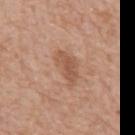* notes: catalogued during a skin exam; not biopsied
* lesion size: ~4 mm (longest diameter)
* acquisition: total-body-photography crop, ~15 mm field of view
* patient: male, roughly 65 years of age
* anatomic site: the abdomen